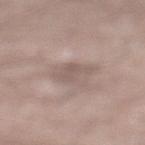Recorded during total-body skin imaging; not selected for excision or biopsy. Captured under white-light illumination. Measured at roughly 3 mm in maximum diameter. A male patient aged approximately 55. An algorithmic analysis of the crop reported an area of roughly 5.5 mm², an outline eccentricity of about 0.65 (0 = round, 1 = elongated), and two-axis asymmetry of about 0.3. And it measured a classifier nevus-likeness of about 0/100 and a detector confidence of about 80 out of 100 that the crop contains a lesion. A close-up tile cropped from a whole-body skin photograph, about 15 mm across. From the leg.